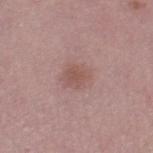lighting: white-light illumination
subject: female, in their mid-40s
image-analysis metrics: an average lesion color of about L≈53 a*≈20 b*≈23 (CIELAB), about 7 CIELAB-L* units darker than the surrounding skin, and a normalized lesion–skin contrast near 6; a border-irregularity index near 2/10
image: 15 mm crop, total-body photography
location: the right thigh